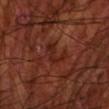Part of a total-body skin-imaging series; this lesion was reviewed on a skin check and was not flagged for biopsy.
A male subject aged 68 to 72.
This is a cross-polarized tile.
The lesion is on the left forearm.
A lesion tile, about 15 mm wide, cut from a 3D total-body photograph.